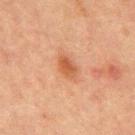Recorded during total-body skin imaging; not selected for excision or biopsy.
Approximately 3.5 mm at its widest.
The subject is a male aged 63 to 67.
Captured under cross-polarized illumination.
A 15 mm close-up tile from a total-body photography series done for melanoma screening.
From the chest.